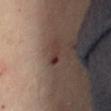Imaged during a routine full-body skin examination; the lesion was not biopsied and no histopathology is available. The lesion is on the lower back. The patient is a female in their 60s. A close-up tile cropped from a whole-body skin photograph, about 15 mm across. The total-body-photography lesion software estimated about 9 CIELAB-L* units darker than the surrounding skin and a lesion-to-skin contrast of about 8.5 (normalized; higher = more distinct). The software also gave internal color variation of about 0 on a 0–10 scale and a peripheral color-asymmetry measure near 0. Measured at roughly 2.5 mm in maximum diameter.